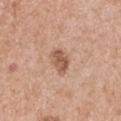{
  "biopsy_status": "not biopsied; imaged during a skin examination",
  "image": {
    "source": "total-body photography crop",
    "field_of_view_mm": 15
  },
  "lesion_size": {
    "long_diameter_mm_approx": 3.0
  },
  "patient": {
    "sex": "male",
    "age_approx": 70
  },
  "site": "right upper arm",
  "lighting": "white-light"
}